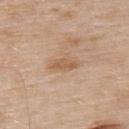follow-up: catalogued during a skin exam; not biopsied
automated metrics: an area of roughly 4 mm², an eccentricity of roughly 0.9, and two-axis asymmetry of about 0.3
patient: male, about 55 years old
image source: ~15 mm tile from a whole-body skin photo
lighting: white-light illumination
size: ≈3.5 mm
body site: the upper back